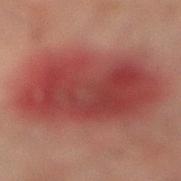Case summary:
* workup: total-body-photography surveillance lesion; no biopsy
* lighting: cross-polarized illumination
* site: the right lower leg
* image-analysis metrics: a border-irregularity rating of about 2/10 and radial color variation of about 1.5
* imaging modality: total-body-photography crop, ~15 mm field of view
* subject: male, aged 73–77
* lesion size: ≈10.5 mm